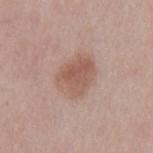<case>
<biopsy_status>not biopsied; imaged during a skin examination</biopsy_status>
<site>mid back</site>
<automated_metrics>
  <area_mm2_approx>13.0</area_mm2_approx>
  <eccentricity>0.65</eccentricity>
  <shape_asymmetry>0.2</shape_asymmetry>
  <nevus_likeness_0_100>50</nevus_likeness_0_100>
</automated_metrics>
<patient>
  <sex>male</sex>
  <age_approx>75</age_approx>
</patient>
<lighting>white-light</lighting>
<lesion_size>
  <long_diameter_mm_approx>4.5</long_diameter_mm_approx>
</lesion_size>
<image>
  <source>total-body photography crop</source>
  <field_of_view_mm>15</field_of_view_mm>
</image>
</case>